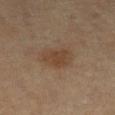Assessment:
The lesion was photographed on a routine skin check and not biopsied; there is no pathology result.
Background:
The recorded lesion diameter is about 4 mm. A lesion tile, about 15 mm wide, cut from a 3D total-body photograph. On the left lower leg. An algorithmic analysis of the crop reported a footprint of about 8.5 mm², an outline eccentricity of about 0.8 (0 = round, 1 = elongated), and a symmetry-axis asymmetry near 0.25. The analysis additionally found a mean CIELAB color near L≈36 a*≈14 b*≈25, roughly 6 lightness units darker than nearby skin, and a normalized border contrast of about 6.5. The subject is a female approximately 55 years of age.